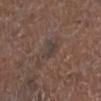This lesion was catalogued during total-body skin photography and was not selected for biopsy. Imaged with white-light lighting. A 15 mm close-up tile from a total-body photography series done for melanoma screening. The subject is a male roughly 75 years of age. Measured at roughly 2.5 mm in maximum diameter. Located on the left lower leg.